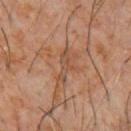Captured during whole-body skin photography for melanoma surveillance; the lesion was not biopsied. Captured under cross-polarized illumination. Cropped from a total-body skin-imaging series; the visible field is about 15 mm. A male patient aged around 60. Located on the chest. Automated tile analysis of the lesion measured a border-irregularity index near 6/10, internal color variation of about 0.5 on a 0–10 scale, and a peripheral color-asymmetry measure near 0.